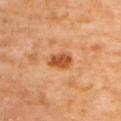Part of a total-body skin-imaging series; this lesion was reviewed on a skin check and was not flagged for biopsy. A 15 mm crop from a total-body photograph taken for skin-cancer surveillance. The total-body-photography lesion software estimated a footprint of about 5 mm², an outline eccentricity of about 0.75 (0 = round, 1 = elongated), and a shape-asymmetry score of about 0.25 (0 = symmetric). The analysis additionally found a border-irregularity rating of about 2.5/10 and a within-lesion color-variation index near 2.5/10. The subject is a female aged 63–67. Captured under cross-polarized illumination. About 3 mm across. The lesion is on the back.